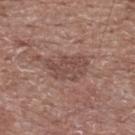Imaged during a routine full-body skin examination; the lesion was not biopsied and no histopathology is available. The subject is a male approximately 60 years of age. This is a white-light tile. A close-up tile cropped from a whole-body skin photograph, about 15 mm across. The lesion is located on the upper back.